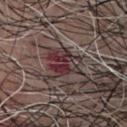Notes:
– notes · imaged on a skin check; not biopsied
– acquisition · 15 mm crop, total-body photography
– subject · male, in their mid- to late 60s
– TBP lesion metrics · an average lesion color of about L≈32 a*≈20 b*≈14 (CIELAB), about 10 CIELAB-L* units darker than the surrounding skin, and a normalized border contrast of about 10; border irregularity of about 5 on a 0–10 scale, a color-variation rating of about 7.5/10, and a peripheral color-asymmetry measure near 2.5
– location · the chest